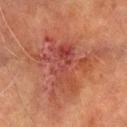Assessment: The lesion was photographed on a routine skin check and not biopsied; there is no pathology result. Clinical summary: The lesion-visualizer software estimated a normalized border contrast of about 7. The software also gave border irregularity of about 8.5 on a 0–10 scale, a color-variation rating of about 5.5/10, and peripheral color asymmetry of about 1.5. It also reported lesion-presence confidence of about 100/100. This image is a 15 mm lesion crop taken from a total-body photograph. From the leg. A male patient, roughly 70 years of age. Measured at roughly 7.5 mm in maximum diameter.